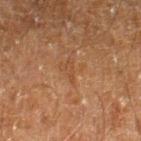Recorded during total-body skin imaging; not selected for excision or biopsy.
A region of skin cropped from a whole-body photographic capture, roughly 15 mm wide.
A male subject aged around 60.
The lesion is on the right lower leg.